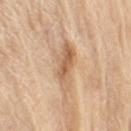Notes:
- workup · imaged on a skin check; not biopsied
- tile lighting · white-light illumination
- image source · 15 mm crop, total-body photography
- subject · male, in their 70s
- site · the right upper arm